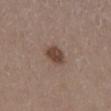Clinical summary:
A female patient, aged around 40. A 15 mm close-up tile from a total-body photography series done for melanoma screening. Located on the left lower leg.